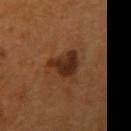Findings:
– acquisition — ~15 mm crop, total-body skin-cancer survey
– patient — male, aged 53–57
– location — the right upper arm